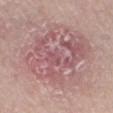Q: Was a biopsy performed?
A: no biopsy performed (imaged during a skin exam)